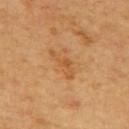Image and clinical context: A male patient aged approximately 65. Captured under cross-polarized illumination. Cropped from a whole-body photographic skin survey; the tile spans about 15 mm. The lesion is on the mid back.